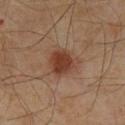No biopsy was performed on this lesion — it was imaged during a full skin examination and was not determined to be concerning. A 15 mm close-up tile from a total-body photography series done for melanoma screening. A male patient, aged 58–62. The lesion is located on the abdomen.